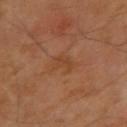Imaged during a routine full-body skin examination; the lesion was not biopsied and no histopathology is available. Imaged with cross-polarized lighting. A roughly 15 mm field-of-view crop from a total-body skin photograph. Automated image analysis of the tile measured an average lesion color of about L≈42 a*≈21 b*≈33 (CIELAB) and a lesion–skin lightness drop of about 5. It also reported border irregularity of about 4 on a 0–10 scale. The lesion is located on the right upper arm. A male patient aged around 70. Measured at roughly 3 mm in maximum diameter.